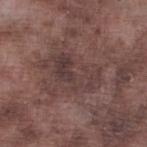Assessment: This lesion was catalogued during total-body skin photography and was not selected for biopsy. Image and clinical context: A male patient approximately 75 years of age. A 15 mm close-up extracted from a 3D total-body photography capture. The lesion is on the left lower leg.